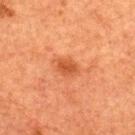Impression: Imaged during a routine full-body skin examination; the lesion was not biopsied and no histopathology is available. Acquisition and patient details: This image is a 15 mm lesion crop taken from a total-body photograph. The patient is a male aged around 50. Approximately 2.5 mm at its widest. On the upper back.